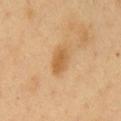Recorded during total-body skin imaging; not selected for excision or biopsy.
A lesion tile, about 15 mm wide, cut from a 3D total-body photograph.
The recorded lesion diameter is about 3.5 mm.
Located on the chest.
Automated image analysis of the tile measured an area of roughly 5.5 mm² and a shape-asymmetry score of about 0.1 (0 = symmetric). It also reported about 10 CIELAB-L* units darker than the surrounding skin and a normalized border contrast of about 7. The software also gave border irregularity of about 2 on a 0–10 scale, internal color variation of about 2 on a 0–10 scale, and peripheral color asymmetry of about 0.5. The analysis additionally found a classifier nevus-likeness of about 5/100 and lesion-presence confidence of about 100/100.
The subject is a male aged approximately 50.